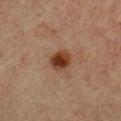Q: Was a biopsy performed?
A: total-body-photography surveillance lesion; no biopsy
Q: How was this image acquired?
A: ~15 mm tile from a whole-body skin photo
Q: What are the patient's age and sex?
A: male, aged 58–62
Q: Illumination type?
A: cross-polarized
Q: What did automated image analysis measure?
A: an area of roughly 5 mm² and an eccentricity of roughly 0.6; an automated nevus-likeness rating near 100 out of 100 and lesion-presence confidence of about 100/100